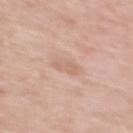workup: imaged on a skin check; not biopsied
site: the upper back
subject: female, aged 38–42
lesion diameter: about 2.5 mm
tile lighting: white-light illumination
acquisition: 15 mm crop, total-body photography